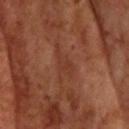The lesion was tiled from a total-body skin photograph and was not biopsied. A male subject, aged 68–72. The tile uses cross-polarized illumination. This image is a 15 mm lesion crop taken from a total-body photograph. The lesion is located on the head or neck. Automated tile analysis of the lesion measured a mean CIELAB color near L≈36 a*≈24 b*≈30 and a normalized lesion–skin contrast near 5. The software also gave an automated nevus-likeness rating near 0 out of 100 and lesion-presence confidence of about 65/100.